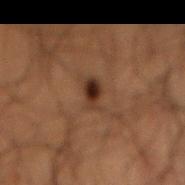Image and clinical context:
The tile uses cross-polarized illumination. Longest diameter approximately 3 mm. The total-body-photography lesion software estimated a lesion area of about 5 mm² and a shape eccentricity near 0.85. It also reported a lesion color around L≈24 a*≈15 b*≈21 in CIELAB, about 8 CIELAB-L* units darker than the surrounding skin, and a normalized lesion–skin contrast near 10. The patient is a male aged around 50. Located on the lower back. A 15 mm close-up extracted from a 3D total-body photography capture.